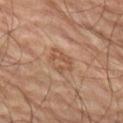Assessment: No biopsy was performed on this lesion — it was imaged during a full skin examination and was not determined to be concerning. Background: From the left thigh. A male subject, about 65 years old. A lesion tile, about 15 mm wide, cut from a 3D total-body photograph.